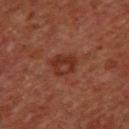Q: Was this lesion biopsied?
A: catalogued during a skin exam; not biopsied
Q: Lesion size?
A: ~3.5 mm (longest diameter)
Q: What is the anatomic site?
A: the chest
Q: Patient demographics?
A: male, aged around 60
Q: Automated lesion metrics?
A: border irregularity of about 2 on a 0–10 scale, a color-variation rating of about 4.5/10, and a peripheral color-asymmetry measure near 1.5
Q: What is the imaging modality?
A: 15 mm crop, total-body photography